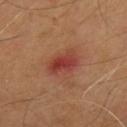Assessment:
Imaged during a routine full-body skin examination; the lesion was not biopsied and no histopathology is available.
Acquisition and patient details:
Located on the chest. A male patient in their mid- to late 50s. This image is a 15 mm lesion crop taken from a total-body photograph.